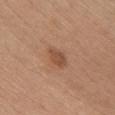The lesion was photographed on a routine skin check and not biopsied; there is no pathology result. A 15 mm close-up extracted from a 3D total-body photography capture. A female subject, aged around 45. The lesion is located on the chest. Longest diameter approximately 3 mm. This is a white-light tile. The lesion-visualizer software estimated a border-irregularity rating of about 2.5/10, a within-lesion color-variation index near 2/10, and a peripheral color-asymmetry measure near 1. The software also gave a classifier nevus-likeness of about 45/100.